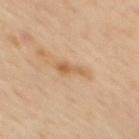diameter: ≈4 mm | imaging modality: 15 mm crop, total-body photography | body site: the back | patient: male, roughly 55 years of age | tile lighting: cross-polarized illumination | image-analysis metrics: a mean CIELAB color near L≈61 a*≈19 b*≈38, roughly 9 lightness units darker than nearby skin, and a normalized border contrast of about 7; border irregularity of about 4.5 on a 0–10 scale, internal color variation of about 2 on a 0–10 scale, and peripheral color asymmetry of about 0.5.